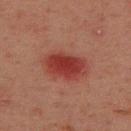Findings:
- workup: no biopsy performed (imaged during a skin exam)
- acquisition: total-body-photography crop, ~15 mm field of view
- lesion size: ~5 mm (longest diameter)
- patient: male, aged 43 to 47
- TBP lesion metrics: a shape-asymmetry score of about 0.15 (0 = symmetric); a lesion color around L≈32 a*≈27 b*≈24 in CIELAB and a lesion–skin lightness drop of about 9; a border-irregularity rating of about 1.5/10 and a color-variation rating of about 3/10; lesion-presence confidence of about 100/100
- site: the back